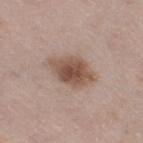Impression: Captured during whole-body skin photography for melanoma surveillance; the lesion was not biopsied. Context: On the right thigh. This image is a 15 mm lesion crop taken from a total-body photograph. A female subject aged 38 to 42.